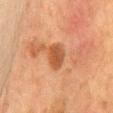biopsy_status: not biopsied; imaged during a skin examination
site: front of the torso
lesion_size:
  long_diameter_mm_approx: 3.5
image:
  source: total-body photography crop
  field_of_view_mm: 15
lighting: cross-polarized
patient:
  sex: female
  age_approx: 50